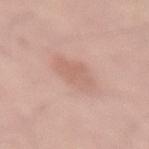A male subject, about 50 years old. A lesion tile, about 15 mm wide, cut from a 3D total-body photograph. From the lower back.